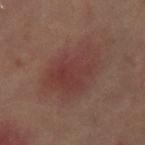Recorded during total-body skin imaging; not selected for excision or biopsy. Approximately 8 mm at its widest. The lesion is on the left thigh. This image is a 15 mm lesion crop taken from a total-body photograph. The patient is a female aged around 50.